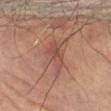size: ≈5 mm
automated lesion analysis: a lesion area of about 9 mm² and a shape-asymmetry score of about 0.35 (0 = symmetric); a mean CIELAB color near L≈45 a*≈21 b*≈24 and a lesion-to-skin contrast of about 6 (normalized; higher = more distinct); border irregularity of about 3.5 on a 0–10 scale, a within-lesion color-variation index near 3.5/10, and peripheral color asymmetry of about 1.5
location: the right leg
patient: female, aged 78–82
tile lighting: cross-polarized
imaging modality: total-body-photography crop, ~15 mm field of view